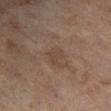The lesion was photographed on a routine skin check and not biopsied; there is no pathology result.
The lesion is located on the right lower leg.
The subject is a female aged approximately 60.
Captured under cross-polarized illumination.
Automated image analysis of the tile measured an area of roughly 3.5 mm² and a shape-asymmetry score of about 0.3 (0 = symmetric). The analysis additionally found a mean CIELAB color near L≈37 a*≈14 b*≈23, a lesion–skin lightness drop of about 5, and a lesion-to-skin contrast of about 5 (normalized; higher = more distinct). The analysis additionally found a classifier nevus-likeness of about 0/100 and a lesion-detection confidence of about 100/100.
A close-up tile cropped from a whole-body skin photograph, about 15 mm across.
The lesion's longest dimension is about 3 mm.